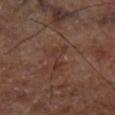Clinical impression:
Imaged during a routine full-body skin examination; the lesion was not biopsied and no histopathology is available.
Background:
A 15 mm close-up tile from a total-body photography series done for melanoma screening. The lesion is located on the left lower leg. Imaged with cross-polarized lighting. Measured at roughly 4 mm in maximum diameter.